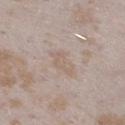patient: female, aged around 25 | image-analysis metrics: a lesion area of about 5 mm², an eccentricity of roughly 0.85, and two-axis asymmetry of about 0.4 | diameter: ≈3.5 mm | imaging modality: total-body-photography crop, ~15 mm field of view | anatomic site: the left lower leg.